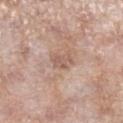Clinical summary: A female patient, about 70 years old. Automated tile analysis of the lesion measured a footprint of about 3.5 mm², a shape eccentricity near 0.75, and a shape-asymmetry score of about 0.3 (0 = symmetric). It also reported an automated nevus-likeness rating near 0 out of 100. A lesion tile, about 15 mm wide, cut from a 3D total-body photograph. About 2.5 mm across. From the right lower leg. The tile uses white-light illumination.